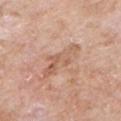{"biopsy_status": "not biopsied; imaged during a skin examination", "patient": {"sex": "male", "age_approx": 60}, "site": "chest", "lighting": "white-light", "automated_metrics": {"eccentricity": 0.9, "shape_asymmetry": 0.45, "nevus_likeness_0_100": 0}, "lesion_size": {"long_diameter_mm_approx": 5.5}, "image": {"source": "total-body photography crop", "field_of_view_mm": 15}}